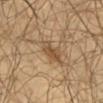Part of a total-body skin-imaging series; this lesion was reviewed on a skin check and was not flagged for biopsy.
The patient is a male about 65 years old.
Captured under cross-polarized illumination.
This image is a 15 mm lesion crop taken from a total-body photograph.
Approximately 3 mm at its widest.
The total-body-photography lesion software estimated an average lesion color of about L≈49 a*≈16 b*≈33 (CIELAB) and a lesion–skin lightness drop of about 9. And it measured internal color variation of about 2 on a 0–10 scale and radial color variation of about 1.